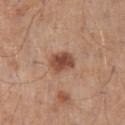Assessment:
Captured during whole-body skin photography for melanoma surveillance; the lesion was not biopsied.
Image and clinical context:
Approximately 3 mm at its widest. Automated tile analysis of the lesion measured a lesion area of about 6.5 mm². The software also gave an average lesion color of about L≈48 a*≈23 b*≈30 (CIELAB), about 13 CIELAB-L* units darker than the surrounding skin, and a lesion-to-skin contrast of about 9.5 (normalized; higher = more distinct). A male subject aged approximately 70. Cropped from a whole-body photographic skin survey; the tile spans about 15 mm. Imaged with white-light lighting. From the lower back.